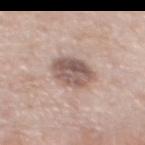Imaged during a routine full-body skin examination; the lesion was not biopsied and no histopathology is available.
The lesion is located on the upper back.
The lesion's longest dimension is about 4 mm.
The subject is a male aged approximately 60.
Cropped from a whole-body photographic skin survey; the tile spans about 15 mm.
Captured under white-light illumination.